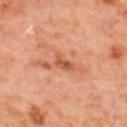Background: Located on the mid back. The patient is a male approximately 60 years of age. A 15 mm close-up extracted from a 3D total-body photography capture.